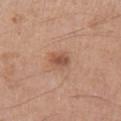<lesion>
<biopsy_status>not biopsied; imaged during a skin examination</biopsy_status>
<image>
  <source>total-body photography crop</source>
  <field_of_view_mm>15</field_of_view_mm>
</image>
<patient>
  <sex>male</sex>
  <age_approx>55</age_approx>
</patient>
<lighting>white-light</lighting>
<lesion_size>
  <long_diameter_mm_approx>2.5</long_diameter_mm_approx>
</lesion_size>
<site>right upper arm</site>
</lesion>